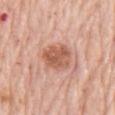The lesion was tiled from a total-body skin photograph and was not biopsied. The lesion is located on the chest. Imaged with white-light lighting. A male subject, aged approximately 80. A 15 mm close-up tile from a total-body photography series done for melanoma screening.